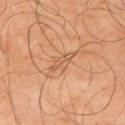Assessment: No biopsy was performed on this lesion — it was imaged during a full skin examination and was not determined to be concerning. Acquisition and patient details: The lesion is located on the left upper arm. The patient is a male in their mid-60s. A 15 mm close-up extracted from a 3D total-body photography capture.